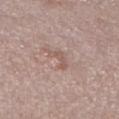{
  "biopsy_status": "not biopsied; imaged during a skin examination",
  "site": "right lower leg",
  "lesion_size": {
    "long_diameter_mm_approx": 3.0
  },
  "lighting": "white-light",
  "image": {
    "source": "total-body photography crop",
    "field_of_view_mm": 15
  },
  "patient": {
    "sex": "male",
    "age_approx": 70
  }
}